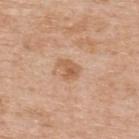| field | value |
|---|---|
| follow-up | total-body-photography surveillance lesion; no biopsy |